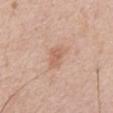| feature | finding |
|---|---|
| notes | catalogued during a skin exam; not biopsied |
| site | the front of the torso |
| subject | male, aged approximately 60 |
| image | 15 mm crop, total-body photography |
| lighting | white-light illumination |
| diameter | ≈3 mm |